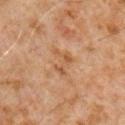{"biopsy_status": "not biopsied; imaged during a skin examination", "image": {"source": "total-body photography crop", "field_of_view_mm": 15}, "patient": {"sex": "male", "age_approx": 60}, "site": "chest", "lesion_size": {"long_diameter_mm_approx": 3.0}}